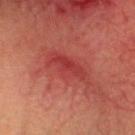This lesion was catalogued during total-body skin photography and was not selected for biopsy.
Cropped from a whole-body photographic skin survey; the tile spans about 15 mm.
A male patient aged 68 to 72.
About 6 mm across.
The lesion is on the head or neck.